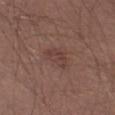workup: total-body-photography surveillance lesion; no biopsy
image-analysis metrics: a lesion area of about 6 mm²; border irregularity of about 3.5 on a 0–10 scale, a within-lesion color-variation index near 2/10, and a peripheral color-asymmetry measure near 1
diameter: ~4 mm (longest diameter)
illumination: white-light
patient: male, in their mid-20s
image source: ~15 mm tile from a whole-body skin photo
anatomic site: the left thigh